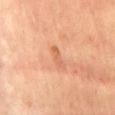• follow-up — no biopsy performed (imaged during a skin exam)
• patient — male, aged 53 to 57
• diameter — about 3 mm
• image source — total-body-photography crop, ~15 mm field of view
• location — the front of the torso
• illumination — cross-polarized
• automated lesion analysis — an eccentricity of roughly 0.95 and a shape-asymmetry score of about 0.4 (0 = symmetric); roughly 7 lightness units darker than nearby skin and a normalized lesion–skin contrast near 5.5; an automated nevus-likeness rating near 0 out of 100